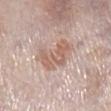Imaged during a routine full-body skin examination; the lesion was not biopsied and no histopathology is available. On the right lower leg. An algorithmic analysis of the crop reported an outline eccentricity of about 0.65 (0 = round, 1 = elongated) and two-axis asymmetry of about 0.45. It also reported a lesion color around L≈61 a*≈19 b*≈26 in CIELAB, a lesion–skin lightness drop of about 9, and a normalized border contrast of about 6.5. And it measured a nevus-likeness score of about 20/100 and a lesion-detection confidence of about 100/100. This image is a 15 mm lesion crop taken from a total-body photograph. The patient is a male aged 58–62. About 4 mm across.